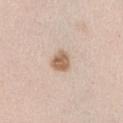Q: Is there a histopathology result?
A: no biopsy performed (imaged during a skin exam)
Q: What did automated image analysis measure?
A: a footprint of about 5.5 mm², an eccentricity of roughly 0.6, and a symmetry-axis asymmetry near 0.3; a mean CIELAB color near L≈62 a*≈17 b*≈30 and about 13 CIELAB-L* units darker than the surrounding skin; a border-irregularity index near 3/10 and a peripheral color-asymmetry measure near 1; an automated nevus-likeness rating near 95 out of 100 and a lesion-detection confidence of about 100/100
Q: How was this image acquired?
A: total-body-photography crop, ~15 mm field of view
Q: Where on the body is the lesion?
A: the left upper arm
Q: Who is the patient?
A: male, aged 43–47
Q: Lesion size?
A: ~3 mm (longest diameter)